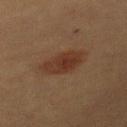No biopsy was performed on this lesion — it was imaged during a full skin examination and was not determined to be concerning. The tile uses cross-polarized illumination. A 15 mm close-up tile from a total-body photography series done for melanoma screening. On the left upper arm. Measured at roughly 6 mm in maximum diameter. A female patient in their 60s.